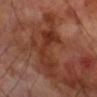Q: Was this lesion biopsied?
A: imaged on a skin check; not biopsied
Q: Lesion location?
A: the right upper arm
Q: What are the patient's age and sex?
A: male, in their 70s
Q: Automated lesion metrics?
A: a border-irregularity index near 9.5/10, a within-lesion color-variation index near 4/10, and a peripheral color-asymmetry measure near 1; a classifier nevus-likeness of about 0/100 and a lesion-detection confidence of about 90/100
Q: What is the imaging modality?
A: total-body-photography crop, ~15 mm field of view
Q: What lighting was used for the tile?
A: cross-polarized illumination